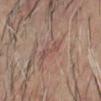The tile uses cross-polarized illumination. A close-up tile cropped from a whole-body skin photograph, about 15 mm across. A male patient aged around 65. The total-body-photography lesion software estimated a mean CIELAB color near L≈50 a*≈19 b*≈23 and a lesion-to-skin contrast of about 5 (normalized; higher = more distinct). And it measured a classifier nevus-likeness of about 0/100 and lesion-presence confidence of about 60/100. Measured at roughly 3.5 mm in maximum diameter. On the head or neck.